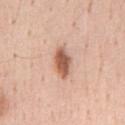– follow-up: total-body-photography surveillance lesion; no biopsy
– diameter: ≈4 mm
– image-analysis metrics: border irregularity of about 2 on a 0–10 scale and a within-lesion color-variation index near 3/10; a nevus-likeness score of about 95/100 and lesion-presence confidence of about 100/100
– subject: male, in their mid-50s
– tile lighting: white-light illumination
– anatomic site: the chest
– image source: 15 mm crop, total-body photography